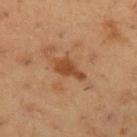– lesion diameter: ~4 mm (longest diameter)
– image-analysis metrics: an area of roughly 6 mm², a shape eccentricity near 0.85, and a shape-asymmetry score of about 0.35 (0 = symmetric); a mean CIELAB color near L≈46 a*≈23 b*≈36 and roughly 10 lightness units darker than nearby skin; a border-irregularity index near 4/10, internal color variation of about 2 on a 0–10 scale, and a peripheral color-asymmetry measure near 0.5
– lighting: cross-polarized illumination
– subject: male, aged 53 to 57
– body site: the left upper arm
– image source: ~15 mm tile from a whole-body skin photo